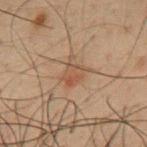Clinical impression:
Captured during whole-body skin photography for melanoma surveillance; the lesion was not biopsied.
Image and clinical context:
A male patient roughly 50 years of age. A 15 mm close-up tile from a total-body photography series done for melanoma screening. From the mid back.